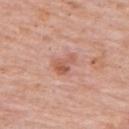workup: imaged on a skin check; not biopsied | automated lesion analysis: a lesion–skin lightness drop of about 10 and a lesion-to-skin contrast of about 7 (normalized; higher = more distinct); border irregularity of about 5 on a 0–10 scale and internal color variation of about 3.5 on a 0–10 scale; a classifier nevus-likeness of about 65/100 | site: the upper back | image: total-body-photography crop, ~15 mm field of view | lesion size: ≈3 mm | illumination: white-light illumination | subject: male, aged approximately 80.